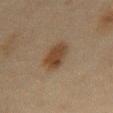This lesion was catalogued during total-body skin photography and was not selected for biopsy.
Imaged with cross-polarized lighting.
An algorithmic analysis of the crop reported an eccentricity of roughly 0.85. And it measured a mean CIELAB color near L≈34 a*≈14 b*≈26, roughly 8 lightness units darker than nearby skin, and a lesion-to-skin contrast of about 8.5 (normalized; higher = more distinct). The software also gave border irregularity of about 2 on a 0–10 scale, a within-lesion color-variation index near 3/10, and radial color variation of about 1.
About 5 mm across.
From the front of the torso.
A close-up tile cropped from a whole-body skin photograph, about 15 mm across.
A male subject approximately 45 years of age.